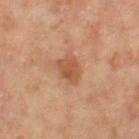Assessment: Imaged during a routine full-body skin examination; the lesion was not biopsied and no histopathology is available.